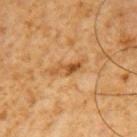Part of a total-body skin-imaging series; this lesion was reviewed on a skin check and was not flagged for biopsy. A 15 mm close-up tile from a total-body photography series done for melanoma screening. This is a cross-polarized tile. A male subject, approximately 60 years of age. An algorithmic analysis of the crop reported a nevus-likeness score of about 0/100 and a lesion-detection confidence of about 90/100. The lesion is located on the left upper arm. Measured at roughly 4.5 mm in maximum diameter.